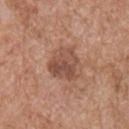The recorded lesion diameter is about 4 mm. On the chest. The tile uses white-light illumination. A close-up tile cropped from a whole-body skin photograph, about 15 mm across. Automated image analysis of the tile measured border irregularity of about 3 on a 0–10 scale, a color-variation rating of about 5/10, and a peripheral color-asymmetry measure near 1.5. The software also gave a nevus-likeness score of about 10/100. A male patient about 65 years old.